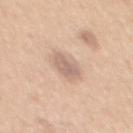notes: imaged on a skin check; not biopsied | patient: male, about 50 years old | size: about 4 mm | illumination: white-light | image-analysis metrics: a border-irregularity index near 3/10 and a color-variation rating of about 2/10 | imaging modality: total-body-photography crop, ~15 mm field of view | location: the mid back.